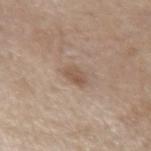This lesion was catalogued during total-body skin photography and was not selected for biopsy.
Cropped from a whole-body photographic skin survey; the tile spans about 15 mm.
The patient is a male aged 68–72.
On the left forearm.